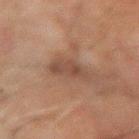biopsy_status: not biopsied; imaged during a skin examination
site: left forearm
patient:
  sex: male
  age_approx: 60
lighting: cross-polarized
lesion_size:
  long_diameter_mm_approx: 4.0
image:
  source: total-body photography crop
  field_of_view_mm: 15